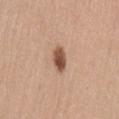notes: total-body-photography surveillance lesion; no biopsy | location: the back | subject: female, in their mid- to late 40s | imaging modality: 15 mm crop, total-body photography.